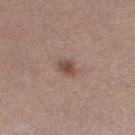The patient is a female aged approximately 30.
From the left lower leg.
A 15 mm crop from a total-body photograph taken for skin-cancer surveillance.
The lesion's longest dimension is about 2.5 mm.
This is a white-light tile.